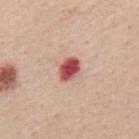– workup: imaged on a skin check; not biopsied
– size: ~3 mm (longest diameter)
– patient: male, in their mid- to late 60s
– automated metrics: a nevus-likeness score of about 0/100 and a detector confidence of about 100 out of 100 that the crop contains a lesion
– image source: ~15 mm crop, total-body skin-cancer survey
– tile lighting: white-light illumination
– body site: the back